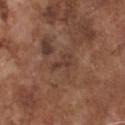Q: Was this lesion biopsied?
A: total-body-photography surveillance lesion; no biopsy
Q: Where on the body is the lesion?
A: the chest
Q: What is the imaging modality?
A: ~15 mm crop, total-body skin-cancer survey
Q: Who is the patient?
A: male, in their mid-70s